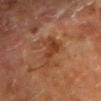biopsy status: imaged on a skin check; not biopsied
diameter: ~5 mm (longest diameter)
patient: male, aged around 65
location: the head or neck
image source: 15 mm crop, total-body photography
tile lighting: cross-polarized
automated metrics: border irregularity of about 6 on a 0–10 scale and a color-variation rating of about 3/10; a nevus-likeness score of about 0/100